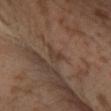| feature | finding |
|---|---|
| patient | male, aged 28–32 |
| anatomic site | the leg |
| acquisition | ~15 mm crop, total-body skin-cancer survey |